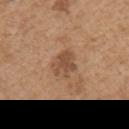Captured during whole-body skin photography for melanoma surveillance; the lesion was not biopsied.
Captured under white-light illumination.
The lesion is located on the chest.
A male patient, approximately 65 years of age.
About 3.5 mm across.
A region of skin cropped from a whole-body photographic capture, roughly 15 mm wide.